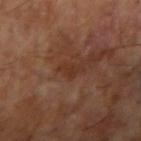{"patient": {"sex": "male", "age_approx": 65}, "image": {"source": "total-body photography crop", "field_of_view_mm": 15}, "site": "right upper arm"}